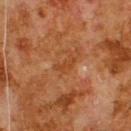follow-up: total-body-photography surveillance lesion; no biopsy
body site: the upper back
imaging modality: total-body-photography crop, ~15 mm field of view
image-analysis metrics: an area of roughly 2.5 mm², a shape eccentricity near 0.95, and a symmetry-axis asymmetry near 0.5; roughly 5 lightness units darker than nearby skin and a normalized border contrast of about 5; a nevus-likeness score of about 0/100 and a detector confidence of about 100 out of 100 that the crop contains a lesion
patient: male, aged approximately 80
size: about 3 mm
lighting: cross-polarized